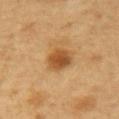Case summary:
- notes — imaged on a skin check; not biopsied
- location — the right forearm
- image — total-body-photography crop, ~15 mm field of view
- illumination — cross-polarized illumination
- patient — female, approximately 45 years of age